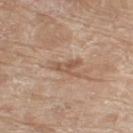follow-up: imaged on a skin check; not biopsied | illumination: white-light | subject: female, in their mid-70s | diameter: ~3 mm (longest diameter) | imaging modality: ~15 mm crop, total-body skin-cancer survey | anatomic site: the right thigh | automated lesion analysis: an area of roughly 3.5 mm² and a symmetry-axis asymmetry near 0.5; border irregularity of about 5 on a 0–10 scale, a within-lesion color-variation index near 1.5/10, and peripheral color asymmetry of about 0.5.